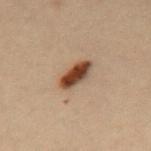The lesion-visualizer software estimated a footprint of about 6 mm², a shape eccentricity near 0.85, and a symmetry-axis asymmetry near 0.15. The software also gave an average lesion color of about L≈35 a*≈18 b*≈26 (CIELAB), roughly 16 lightness units darker than nearby skin, and a lesion-to-skin contrast of about 13.5 (normalized; higher = more distinct). And it measured a nevus-likeness score of about 100/100. A region of skin cropped from a whole-body photographic capture, roughly 15 mm wide. A female subject, aged approximately 30. On the back.